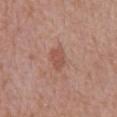Q: Was this lesion biopsied?
A: imaged on a skin check; not biopsied
Q: Who is the patient?
A: male, in their mid- to late 50s
Q: Where on the body is the lesion?
A: the mid back
Q: How large is the lesion?
A: ≈3 mm
Q: How was the tile lit?
A: white-light illumination
Q: What is the imaging modality?
A: total-body-photography crop, ~15 mm field of view